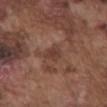{
  "biopsy_status": "not biopsied; imaged during a skin examination",
  "lesion_size": {
    "long_diameter_mm_approx": 2.5
  },
  "lighting": "white-light",
  "site": "abdomen",
  "patient": {
    "sex": "male",
    "age_approx": 75
  },
  "image": {
    "source": "total-body photography crop",
    "field_of_view_mm": 15
  },
  "automated_metrics": {
    "area_mm2_approx": 4.0,
    "eccentricity": 0.7,
    "shape_asymmetry": 0.3,
    "border_irregularity_0_10": 3.0,
    "color_variation_0_10": 1.5,
    "peripheral_color_asymmetry": 0.5
  }
}